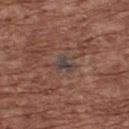Impression:
The lesion was tiled from a total-body skin photograph and was not biopsied.
Image and clinical context:
The lesion's longest dimension is about 3 mm. The patient is a male aged 73–77. The tile uses white-light illumination. On the upper back. The total-body-photography lesion software estimated an area of roughly 4 mm², a shape eccentricity near 0.75, and a shape-asymmetry score of about 0.45 (0 = symmetric). It also reported an average lesion color of about L≈40 a*≈11 b*≈18 (CIELAB) and a lesion-to-skin contrast of about 8 (normalized; higher = more distinct). It also reported a lesion-detection confidence of about 70/100. A 15 mm crop from a total-body photograph taken for skin-cancer surveillance.